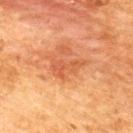workup = catalogued during a skin exam; not biopsied
subject = male, aged 48–52
body site = the upper back
size = about 4 mm
illumination = cross-polarized illumination
image source = 15 mm crop, total-body photography
image-analysis metrics = a lesion area of about 5.5 mm² and a symmetry-axis asymmetry near 0.45; a mean CIELAB color near L≈47 a*≈26 b*≈35; a border-irregularity index near 6/10, internal color variation of about 2 on a 0–10 scale, and radial color variation of about 0.5; a classifier nevus-likeness of about 10/100 and a lesion-detection confidence of about 100/100